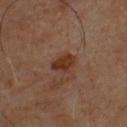location=the front of the torso; lesion size=~3 mm (longest diameter); imaging modality=~15 mm tile from a whole-body skin photo; lighting=cross-polarized; patient=male, in their 50s.